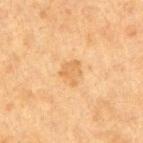  biopsy_status: not biopsied; imaged during a skin examination
  image:
    source: total-body photography crop
    field_of_view_mm: 15
  lighting: cross-polarized
  lesion_size:
    long_diameter_mm_approx: 2.5
  patient:
    sex: female
    age_approx: 40
  site: arm